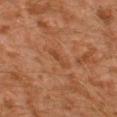Clinical impression: Captured during whole-body skin photography for melanoma surveillance; the lesion was not biopsied. Image and clinical context: The patient is a male in their 30s. This image is a 15 mm lesion crop taken from a total-body photograph. The lesion is on the left thigh. About 2.5 mm across.